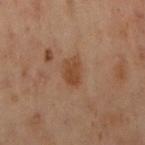Assessment:
Part of a total-body skin-imaging series; this lesion was reviewed on a skin check and was not flagged for biopsy.
Acquisition and patient details:
The tile uses cross-polarized illumination. The lesion's longest dimension is about 3 mm. Automated tile analysis of the lesion measured an average lesion color of about L≈37 a*≈18 b*≈27 (CIELAB), a lesion–skin lightness drop of about 7, and a normalized lesion–skin contrast near 7. The software also gave a detector confidence of about 100 out of 100 that the crop contains a lesion. This image is a 15 mm lesion crop taken from a total-body photograph. The lesion is on the left arm. The patient is a female in their mid-50s.